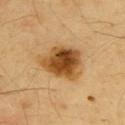automated lesion analysis: a footprint of about 18 mm², an outline eccentricity of about 0.65 (0 = round, 1 = elongated), and two-axis asymmetry of about 0.25; border irregularity of about 2.5 on a 0–10 scale, internal color variation of about 8 on a 0–10 scale, and radial color variation of about 2 | image source: total-body-photography crop, ~15 mm field of view | subject: male, in their mid-60s | anatomic site: the upper back | lighting: cross-polarized illumination | lesion diameter: ~5.5 mm (longest diameter).